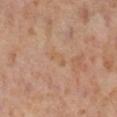The lesion was tiled from a total-body skin photograph and was not biopsied. From the right lower leg. The subject is a female about 55 years old. This is a cross-polarized tile. Automated tile analysis of the lesion measured an outline eccentricity of about 0.9 (0 = round, 1 = elongated) and a shape-asymmetry score of about 0.45 (0 = symmetric). It also reported a mean CIELAB color near L≈58 a*≈19 b*≈34, a lesion–skin lightness drop of about 4, and a normalized lesion–skin contrast near 4.5. The analysis additionally found border irregularity of about 5 on a 0–10 scale and radial color variation of about 0. Cropped from a total-body skin-imaging series; the visible field is about 15 mm.